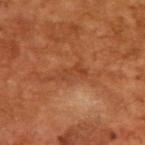The lesion was photographed on a routine skin check and not biopsied; there is no pathology result. Captured under cross-polarized illumination. A male subject, about 65 years old. Approximately 3.5 mm at its widest. Cropped from a whole-body photographic skin survey; the tile spans about 15 mm.